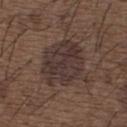| key | value |
|---|---|
| follow-up | no biopsy performed (imaged during a skin exam) |
| imaging modality | 15 mm crop, total-body photography |
| tile lighting | white-light |
| body site | the back |
| diameter | ≈6.5 mm |
| subject | male, aged 48 to 52 |
| image-analysis metrics | border irregularity of about 3 on a 0–10 scale and a within-lesion color-variation index near 3.5/10; a classifier nevus-likeness of about 10/100 and a lesion-detection confidence of about 95/100 |